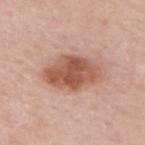Part of a total-body skin-imaging series; this lesion was reviewed on a skin check and was not flagged for biopsy. A male subject in their 60s. Located on the upper back. A close-up tile cropped from a whole-body skin photograph, about 15 mm across.